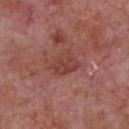Q: Automated lesion metrics?
A: an average lesion color of about L≈42 a*≈25 b*≈26 (CIELAB) and a normalized border contrast of about 6; a detector confidence of about 100 out of 100 that the crop contains a lesion
Q: What is the lesion's diameter?
A: ~3.5 mm (longest diameter)
Q: Who is the patient?
A: male, about 65 years old
Q: Where on the body is the lesion?
A: the chest
Q: How was the tile lit?
A: white-light illumination
Q: What kind of image is this?
A: total-body-photography crop, ~15 mm field of view